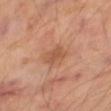notes — catalogued during a skin exam; not biopsied
image — ~15 mm tile from a whole-body skin photo
anatomic site — the leg
diameter — about 3 mm
patient — male, roughly 70 years of age